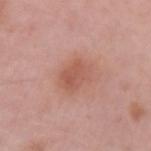| key | value |
|---|---|
| workup | imaged on a skin check; not biopsied |
| image | total-body-photography crop, ~15 mm field of view |
| lighting | white-light illumination |
| diameter | ≈4 mm |
| subject | female, roughly 45 years of age |
| site | the left upper arm |